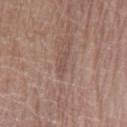Part of a total-body skin-imaging series; this lesion was reviewed on a skin check and was not flagged for biopsy. Cropped from a total-body skin-imaging series; the visible field is about 15 mm. Approximately 2.5 mm at its widest. Automated image analysis of the tile measured an average lesion color of about L≈49 a*≈17 b*≈24 (CIELAB), about 7 CIELAB-L* units darker than the surrounding skin, and a normalized lesion–skin contrast near 5. The software also gave a classifier nevus-likeness of about 0/100. Captured under white-light illumination. A male subject roughly 70 years of age. On the left lower leg.